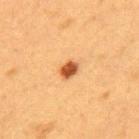<record>
  <biopsy_status>not biopsied; imaged during a skin examination</biopsy_status>
  <automated_metrics>
    <area_mm2_approx>4.0</area_mm2_approx>
    <eccentricity>0.7</eccentricity>
    <shape_asymmetry>0.25</shape_asymmetry>
    <nevus_likeness_0_100>100</nevus_likeness_0_100>
    <lesion_detection_confidence_0_100>100</lesion_detection_confidence_0_100>
  </automated_metrics>
  <image>
    <source>total-body photography crop</source>
    <field_of_view_mm>15</field_of_view_mm>
  </image>
  <lighting>cross-polarized</lighting>
  <patient>
    <sex>female</sex>
    <age_approx>40</age_approx>
  </patient>
  <site>mid back</site>
</record>